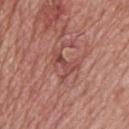patient:
  sex: male
  age_approx: 75
image:
  source: total-body photography crop
  field_of_view_mm: 15
site: upper back